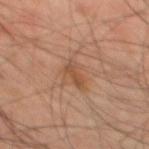| feature | finding |
|---|---|
| notes | catalogued during a skin exam; not biopsied |
| site | the mid back |
| imaging modality | 15 mm crop, total-body photography |
| tile lighting | cross-polarized illumination |
| automated lesion analysis | a classifier nevus-likeness of about 0/100 and a detector confidence of about 100 out of 100 that the crop contains a lesion |
| subject | male, aged approximately 45 |
| lesion diameter | about 3.5 mm |